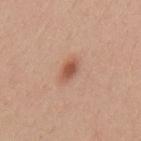{
  "site": "mid back",
  "image": {
    "source": "total-body photography crop",
    "field_of_view_mm": 15
  },
  "lighting": "white-light",
  "lesion_size": {
    "long_diameter_mm_approx": 2.5
  },
  "patient": {
    "sex": "male",
    "age_approx": 30
  }
}